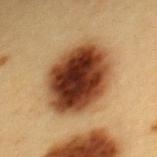Case summary:
• biopsy status: no biopsy performed (imaged during a skin exam)
• illumination: cross-polarized illumination
• subject: female, aged 28 to 32
• image source: total-body-photography crop, ~15 mm field of view
• location: the upper back
• size: about 7.5 mm